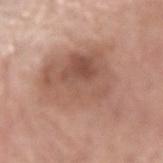Impression:
No biopsy was performed on this lesion — it was imaged during a full skin examination and was not determined to be concerning.
Clinical summary:
Captured under white-light illumination. The recorded lesion diameter is about 8.5 mm. Located on the arm. The subject is a male aged around 70. A roughly 15 mm field-of-view crop from a total-body skin photograph.